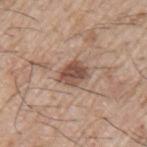The lesion was photographed on a routine skin check and not biopsied; there is no pathology result.
The total-body-photography lesion software estimated a footprint of about 7 mm², an outline eccentricity of about 0.65 (0 = round, 1 = elongated), and a shape-asymmetry score of about 0.2 (0 = symmetric). It also reported a mean CIELAB color near L≈50 a*≈19 b*≈26 and a lesion-to-skin contrast of about 9 (normalized; higher = more distinct). The analysis additionally found a nevus-likeness score of about 85/100.
A male patient, in their mid-60s.
Approximately 3.5 mm at its widest.
Located on the left upper arm.
A region of skin cropped from a whole-body photographic capture, roughly 15 mm wide.
Captured under white-light illumination.